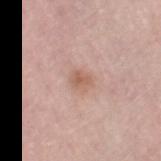Clinical impression: The lesion was tiled from a total-body skin photograph and was not biopsied. Context: Measured at roughly 2.5 mm in maximum diameter. The subject is a female roughly 65 years of age. The tile uses white-light illumination. A close-up tile cropped from a whole-body skin photograph, about 15 mm across. The total-body-photography lesion software estimated a mean CIELAB color near L≈60 a*≈21 b*≈28, about 8 CIELAB-L* units darker than the surrounding skin, and a normalized lesion–skin contrast near 6.5. The analysis additionally found a classifier nevus-likeness of about 10/100. From the right thigh.